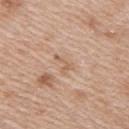Case summary:
• workup · catalogued during a skin exam; not biopsied
• location · the arm
• imaging modality · 15 mm crop, total-body photography
• illumination · white-light illumination
• lesion diameter · ~2.5 mm (longest diameter)
• subject · female, about 40 years old